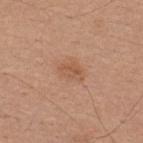No biopsy was performed on this lesion — it was imaged during a full skin examination and was not determined to be concerning. The lesion is located on the upper back. A 15 mm close-up extracted from a 3D total-body photography capture. The subject is a male aged 28–32.